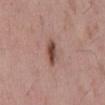<record>
<biopsy_status>not biopsied; imaged during a skin examination</biopsy_status>
<lighting>white-light</lighting>
<automated_metrics>
  <area_mm2_approx>4.5</area_mm2_approx>
  <eccentricity>0.9</eccentricity>
  <shape_asymmetry>0.35</shape_asymmetry>
  <cielab_L>47</cielab_L>
  <cielab_a>21</cielab_a>
  <cielab_b>24</cielab_b>
  <vs_skin_darker_L>13.0</vs_skin_darker_L>
  <vs_skin_contrast_norm>10.0</vs_skin_contrast_norm>
</automated_metrics>
<image>
  <source>total-body photography crop</source>
  <field_of_view_mm>15</field_of_view_mm>
</image>
<lesion_size>
  <long_diameter_mm_approx>3.5</long_diameter_mm_approx>
</lesion_size>
<patient>
  <sex>male</sex>
  <age_approx>55</age_approx>
</patient>
<site>mid back</site>
</record>